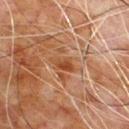Imaged during a routine full-body skin examination; the lesion was not biopsied and no histopathology is available.
The patient is a male aged 78–82.
Cropped from a total-body skin-imaging series; the visible field is about 15 mm.
On the chest.